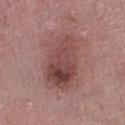The lesion was photographed on a routine skin check and not biopsied; there is no pathology result. A female subject aged 63 to 67. A lesion tile, about 15 mm wide, cut from a 3D total-body photograph. On the left lower leg. Imaged with white-light lighting. About 7.5 mm across. Automated tile analysis of the lesion measured a footprint of about 29 mm², a shape eccentricity near 0.75, and two-axis asymmetry of about 0.25. It also reported a classifier nevus-likeness of about 80/100 and a lesion-detection confidence of about 100/100.